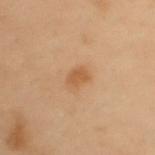| feature | finding |
|---|---|
| biopsy status | total-body-photography surveillance lesion; no biopsy |
| lighting | cross-polarized illumination |
| image source | ~15 mm tile from a whole-body skin photo |
| patient | female, aged approximately 60 |
| automated lesion analysis | a symmetry-axis asymmetry near 0.2; a mean CIELAB color near L≈49 a*≈19 b*≈34, about 8 CIELAB-L* units darker than the surrounding skin, and a normalized border contrast of about 6.5; a border-irregularity rating of about 2/10, a color-variation rating of about 1/10, and peripheral color asymmetry of about 0.5; lesion-presence confidence of about 100/100 |
| site | the upper back |
| diameter | about 2.5 mm |